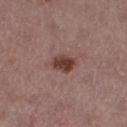Clinical impression: Recorded during total-body skin imaging; not selected for excision or biopsy. Clinical summary: The lesion is located on the right thigh. A 15 mm crop from a total-body photograph taken for skin-cancer surveillance. A female patient aged 53–57. Measured at roughly 3 mm in maximum diameter. This is a white-light tile. The total-body-photography lesion software estimated an average lesion color of about L≈40 a*≈21 b*≈23 (CIELAB), about 13 CIELAB-L* units darker than the surrounding skin, and a normalized lesion–skin contrast near 10.5.